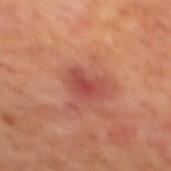Assessment:
Part of a total-body skin-imaging series; this lesion was reviewed on a skin check and was not flagged for biopsy.
Background:
The subject is a male aged around 70. Automated image analysis of the tile measured a mean CIELAB color near L≈47 a*≈31 b*≈28, about 8 CIELAB-L* units darker than the surrounding skin, and a lesion-to-skin contrast of about 6.5 (normalized; higher = more distinct). And it measured a border-irregularity index near 3.5/10 and a peripheral color-asymmetry measure near 1. About 3.5 mm across. Captured under cross-polarized illumination. A roughly 15 mm field-of-view crop from a total-body skin photograph. On the mid back.